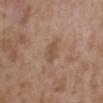Q: Was a biopsy performed?
A: imaged on a skin check; not biopsied
Q: What is the anatomic site?
A: the upper back
Q: Patient demographics?
A: female, approximately 35 years of age
Q: How was this image acquired?
A: ~15 mm tile from a whole-body skin photo